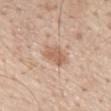location = the back; patient = male, aged around 55; image = ~15 mm tile from a whole-body skin photo.